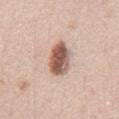Imaged during a routine full-body skin examination; the lesion was not biopsied and no histopathology is available. A 15 mm close-up tile from a total-body photography series done for melanoma screening. A male patient aged 48–52. The lesion is on the chest. Automated image analysis of the tile measured an area of roughly 10 mm², a shape eccentricity near 0.85, and a symmetry-axis asymmetry near 0.2. It also reported roughly 18 lightness units darker than nearby skin and a lesion-to-skin contrast of about 11 (normalized; higher = more distinct). The analysis additionally found internal color variation of about 6.5 on a 0–10 scale and a peripheral color-asymmetry measure near 2.